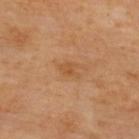{
  "biopsy_status": "not biopsied; imaged during a skin examination",
  "site": "upper back",
  "image": {
    "source": "total-body photography crop",
    "field_of_view_mm": 15
  }
}